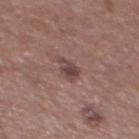Notes:
* subject · male, aged approximately 55
* image · 15 mm crop, total-body photography
* automated metrics · a lesion color around L≈43 a*≈19 b*≈19 in CIELAB and a normalized border contrast of about 7.5; a within-lesion color-variation index near 2.5/10; a classifier nevus-likeness of about 10/100
* body site · the chest
* tile lighting · white-light illumination